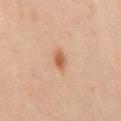notes — total-body-photography surveillance lesion; no biopsy
site — the mid back
lesion diameter — ~3 mm (longest diameter)
patient — male, approximately 45 years of age
lighting — cross-polarized illumination
image — total-body-photography crop, ~15 mm field of view